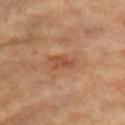Imaged during a routine full-body skin examination; the lesion was not biopsied and no histopathology is available. Longest diameter approximately 3 mm. A lesion tile, about 15 mm wide, cut from a 3D total-body photograph. A female subject about 75 years old. Imaged with cross-polarized lighting. Automated image analysis of the tile measured a nevus-likeness score of about 0/100. On the left upper arm.